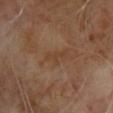Clinical impression: Part of a total-body skin-imaging series; this lesion was reviewed on a skin check and was not flagged for biopsy. Context: The subject is a male approximately 65 years of age. Located on the chest. Imaged with cross-polarized lighting. Cropped from a total-body skin-imaging series; the visible field is about 15 mm.